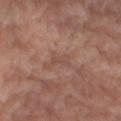Assessment: The lesion was photographed on a routine skin check and not biopsied; there is no pathology result. Context: The total-body-photography lesion software estimated an area of roughly 3 mm², a shape eccentricity near 0.9, and a symmetry-axis asymmetry near 0.45. It also reported a lesion color around L≈49 a*≈21 b*≈25 in CIELAB, about 6 CIELAB-L* units darker than the surrounding skin, and a normalized lesion–skin contrast near 4.5. And it measured a lesion-detection confidence of about 75/100. The patient is a female in their 60s. The lesion is on the right lower leg. Captured under cross-polarized illumination. The recorded lesion diameter is about 2.5 mm. A 15 mm close-up extracted from a 3D total-body photography capture.